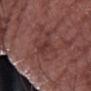Q: Was this lesion biopsied?
A: no biopsy performed (imaged during a skin exam)